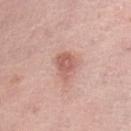{"biopsy_status": "not biopsied; imaged during a skin examination", "lesion_size": {"long_diameter_mm_approx": 3.5}, "lighting": "white-light", "patient": {"sex": "female", "age_approx": 65}, "image": {"source": "total-body photography crop", "field_of_view_mm": 15}, "site": "left lower leg"}